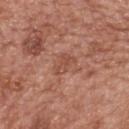<case>
<biopsy_status>not biopsied; imaged during a skin examination</biopsy_status>
<site>back</site>
<patient>
  <sex>female</sex>
  <age_approx>60</age_approx>
</patient>
<image>
  <source>total-body photography crop</source>
  <field_of_view_mm>15</field_of_view_mm>
</image>
<lighting>white-light</lighting>
<lesion_size>
  <long_diameter_mm_approx>3.0</long_diameter_mm_approx>
</lesion_size>
</case>